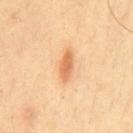notes: imaged on a skin check; not biopsied
patient: male, roughly 40 years of age
lighting: cross-polarized illumination
image: ~15 mm tile from a whole-body skin photo
anatomic site: the chest
lesion diameter: ≈3.5 mm